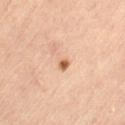| key | value |
|---|---|
| workup | catalogued during a skin exam; not biopsied |
| acquisition | ~15 mm crop, total-body skin-cancer survey |
| diameter | ~2.5 mm (longest diameter) |
| body site | the left thigh |
| subject | female, in their 60s |